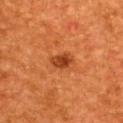Imaged during a routine full-body skin examination; the lesion was not biopsied and no histopathology is available.
From the back.
A region of skin cropped from a whole-body photographic capture, roughly 15 mm wide.
Captured under cross-polarized illumination.
The subject is a male in their mid- to late 40s.
Automated tile analysis of the lesion measured two-axis asymmetry of about 0.3. The software also gave a border-irregularity index near 2.5/10, internal color variation of about 3.5 on a 0–10 scale, and radial color variation of about 1.5. The analysis additionally found a classifier nevus-likeness of about 95/100 and a lesion-detection confidence of about 100/100.
Approximately 2.5 mm at its widest.